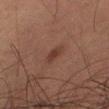The lesion was tiled from a total-body skin photograph and was not biopsied. The lesion is located on the left thigh. Longest diameter approximately 3 mm. An algorithmic analysis of the crop reported a lesion area of about 3 mm² and two-axis asymmetry of about 0.25. The analysis additionally found a normalized border contrast of about 7. The analysis additionally found border irregularity of about 3 on a 0–10 scale, internal color variation of about 0.5 on a 0–10 scale, and radial color variation of about 0. It also reported a detector confidence of about 100 out of 100 that the crop contains a lesion. A male patient aged around 65. This is a cross-polarized tile. A region of skin cropped from a whole-body photographic capture, roughly 15 mm wide.